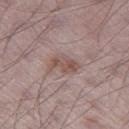| field | value |
|---|---|
| biopsy status | imaged on a skin check; not biopsied |
| site | the left thigh |
| image source | total-body-photography crop, ~15 mm field of view |
| diameter | about 3.5 mm |
| patient | male, aged around 70 |
| image-analysis metrics | a symmetry-axis asymmetry near 0.3; a border-irregularity index near 3.5/10 and a within-lesion color-variation index near 3.5/10 |